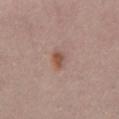Q: Is there a histopathology result?
A: no biopsy performed (imaged during a skin exam)
Q: What is the imaging modality?
A: total-body-photography crop, ~15 mm field of view
Q: Lesion size?
A: about 2.5 mm
Q: What lighting was used for the tile?
A: white-light illumination
Q: Lesion location?
A: the chest
Q: Who is the patient?
A: female, in their 40s